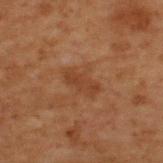Captured during whole-body skin photography for melanoma surveillance; the lesion was not biopsied.